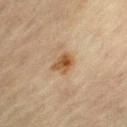On the right lower leg.
The subject is a female aged approximately 65.
About 3 mm across.
The lesion-visualizer software estimated a lesion–skin lightness drop of about 10 and a normalized border contrast of about 9.
Captured under cross-polarized illumination.
Cropped from a whole-body photographic skin survey; the tile spans about 15 mm.